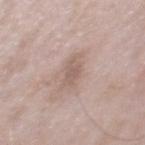  biopsy_status: not biopsied; imaged during a skin examination
  site: mid back
  patient:
    sex: male
    age_approx: 55
  image:
    source: total-body photography crop
    field_of_view_mm: 15